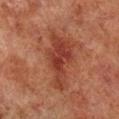Imaged during a routine full-body skin examination; the lesion was not biopsied and no histopathology is available. Longest diameter approximately 6.5 mm. The patient is a male aged around 70. On the right lower leg. This is a cross-polarized tile. A close-up tile cropped from a whole-body skin photograph, about 15 mm across. The lesion-visualizer software estimated a lesion area of about 16 mm², a shape eccentricity near 0.9, and a shape-asymmetry score of about 0.4 (0 = symmetric). And it measured border irregularity of about 5.5 on a 0–10 scale, a within-lesion color-variation index near 3.5/10, and radial color variation of about 1. The software also gave an automated nevus-likeness rating near 15 out of 100 and a lesion-detection confidence of about 100/100.